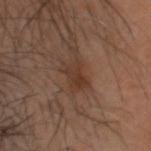biopsy status=total-body-photography surveillance lesion; no biopsy
lesion size=≈3.5 mm
automated metrics=a lesion area of about 6.5 mm², an eccentricity of roughly 0.7, and two-axis asymmetry of about 0.2; a border-irregularity index near 3/10, internal color variation of about 3 on a 0–10 scale, and peripheral color asymmetry of about 1
tile lighting=cross-polarized
image=~15 mm tile from a whole-body skin photo
location=the head or neck
patient=male, aged around 45